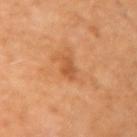| field | value |
|---|---|
| workup | imaged on a skin check; not biopsied |
| image | 15 mm crop, total-body photography |
| size | about 3 mm |
| location | the right upper arm |
| automated lesion analysis | a border-irregularity index near 2.5/10, a color-variation rating of about 1.5/10, and a peripheral color-asymmetry measure near 0.5; an automated nevus-likeness rating near 15 out of 100 and a lesion-detection confidence of about 100/100 |
| subject | female, about 50 years old |